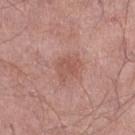notes: catalogued during a skin exam; not biopsied
size: about 3.5 mm
subject: male, approximately 55 years of age
anatomic site: the right lower leg
illumination: white-light
image source: ~15 mm crop, total-body skin-cancer survey
automated metrics: a lesion area of about 6.5 mm² and an eccentricity of roughly 0.6; border irregularity of about 4 on a 0–10 scale, internal color variation of about 2.5 on a 0–10 scale, and a peripheral color-asymmetry measure near 1